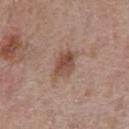Assessment:
This lesion was catalogued during total-body skin photography and was not selected for biopsy.
Acquisition and patient details:
A male patient approximately 70 years of age. Located on the chest. A 15 mm close-up extracted from a 3D total-body photography capture. An algorithmic analysis of the crop reported a nevus-likeness score of about 75/100 and lesion-presence confidence of about 100/100. This is a white-light tile. About 4 mm across.